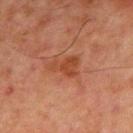| field | value |
|---|---|
| follow-up | imaged on a skin check; not biopsied |
| TBP lesion metrics | a footprint of about 6 mm², an eccentricity of roughly 0.75, and a symmetry-axis asymmetry near 0.5; a nevus-likeness score of about 5/100 and a detector confidence of about 100 out of 100 that the crop contains a lesion |
| patient | male, aged 63–67 |
| image | total-body-photography crop, ~15 mm field of view |
| anatomic site | the front of the torso |
| lesion size | about 4 mm |